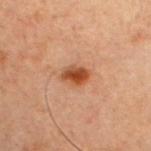The subject is a male aged approximately 60.
Captured under cross-polarized illumination.
Approximately 3 mm at its widest.
Cropped from a whole-body photographic skin survey; the tile spans about 15 mm.
An algorithmic analysis of the crop reported a lesion area of about 5.5 mm² and a shape eccentricity near 0.75. The software also gave a mean CIELAB color near L≈37 a*≈22 b*≈30, about 11 CIELAB-L* units darker than the surrounding skin, and a lesion-to-skin contrast of about 10 (normalized; higher = more distinct). The analysis additionally found a border-irregularity index near 2/10.
On the chest.